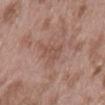A male subject in their mid-50s. On the lower back. A close-up tile cropped from a whole-body skin photograph, about 15 mm across.